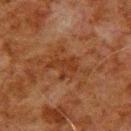notes: imaged on a skin check; not biopsied
tile lighting: cross-polarized illumination
image: ~15 mm tile from a whole-body skin photo
subject: male, aged 78 to 82
anatomic site: the upper back
TBP lesion metrics: a mean CIELAB color near L≈29 a*≈20 b*≈28, roughly 6 lightness units darker than nearby skin, and a lesion-to-skin contrast of about 6 (normalized; higher = more distinct); a border-irregularity index near 9/10, internal color variation of about 2.5 on a 0–10 scale, and a peripheral color-asymmetry measure near 1; an automated nevus-likeness rating near 0 out of 100 and a detector confidence of about 100 out of 100 that the crop contains a lesion
lesion size: ≈4.5 mm